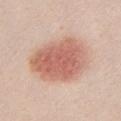Notes:
• subject: female, aged approximately 20
• body site: the chest
• image: total-body-photography crop, ~15 mm field of view